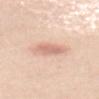- image — ~15 mm crop, total-body skin-cancer survey
- site — the back
- subject — female, approximately 45 years of age